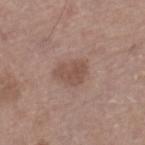Q: Was a biopsy performed?
A: total-body-photography surveillance lesion; no biopsy
Q: Lesion size?
A: ≈3.5 mm
Q: What is the anatomic site?
A: the left lower leg
Q: Who is the patient?
A: male, roughly 65 years of age
Q: Automated lesion metrics?
A: a within-lesion color-variation index near 2/10 and peripheral color asymmetry of about 0.5; an automated nevus-likeness rating near 5 out of 100
Q: What is the imaging modality?
A: total-body-photography crop, ~15 mm field of view
Q: How was the tile lit?
A: white-light illumination